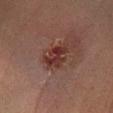<tbp_lesion>
  <image>
    <source>total-body photography crop</source>
    <field_of_view_mm>15</field_of_view_mm>
  </image>
  <patient>
    <sex>female</sex>
    <age_approx>40</age_approx>
  </patient>
  <site>right lower leg</site>
</tbp_lesion>